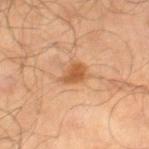Clinical impression: No biopsy was performed on this lesion — it was imaged during a full skin examination and was not determined to be concerning. Acquisition and patient details: Measured at roughly 3 mm in maximum diameter. This is a cross-polarized tile. A male subject in their mid-60s. A roughly 15 mm field-of-view crop from a total-body skin photograph. The total-body-photography lesion software estimated an outline eccentricity of about 0.65 (0 = round, 1 = elongated) and a symmetry-axis asymmetry near 0.3. The software also gave a color-variation rating of about 3/10 and peripheral color asymmetry of about 1. From the right lower leg.